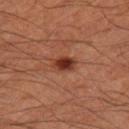Background:
On the right thigh. A male patient approximately 60 years of age. A close-up tile cropped from a whole-body skin photograph, about 15 mm across.